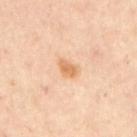Image and clinical context:
The subject is roughly 55 years of age. Imaged with cross-polarized lighting. A roughly 15 mm field-of-view crop from a total-body skin photograph. From the upper back.